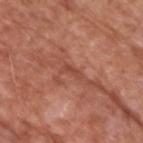Recorded during total-body skin imaging; not selected for excision or biopsy. Measured at roughly 2.5 mm in maximum diameter. A male subject about 75 years old. On the right upper arm. The total-body-photography lesion software estimated a mean CIELAB color near L≈47 a*≈27 b*≈31 and a lesion–skin lightness drop of about 7. The software also gave a border-irregularity rating of about 5/10, internal color variation of about 0 on a 0–10 scale, and a peripheral color-asymmetry measure near 0. The software also gave an automated nevus-likeness rating near 0 out of 100 and a lesion-detection confidence of about 65/100. Captured under white-light illumination. This image is a 15 mm lesion crop taken from a total-body photograph.